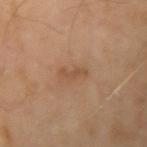notes=catalogued during a skin exam; not biopsied